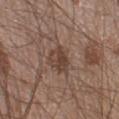notes: imaged on a skin check; not biopsied
imaging modality: total-body-photography crop, ~15 mm field of view
patient: male, about 20 years old
anatomic site: the left lower leg